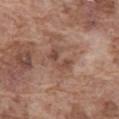Clinical impression:
Captured during whole-body skin photography for melanoma surveillance; the lesion was not biopsied.
Image and clinical context:
The tile uses white-light illumination. Approximately 3.5 mm at its widest. The subject is a male in their mid- to late 70s. Cropped from a total-body skin-imaging series; the visible field is about 15 mm. The lesion is on the abdomen. Automated tile analysis of the lesion measured a lesion area of about 5 mm², a shape eccentricity near 0.85, and a symmetry-axis asymmetry near 0.45. It also reported a lesion color around L≈47 a*≈20 b*≈25 in CIELAB and a lesion-to-skin contrast of about 6.5 (normalized; higher = more distinct). It also reported a border-irregularity rating of about 6.5/10, a within-lesion color-variation index near 2/10, and peripheral color asymmetry of about 0.5.